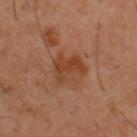Q: Was a biopsy performed?
A: imaged on a skin check; not biopsied
Q: Who is the patient?
A: male, aged approximately 40
Q: Where on the body is the lesion?
A: the upper back
Q: What kind of image is this?
A: ~15 mm crop, total-body skin-cancer survey